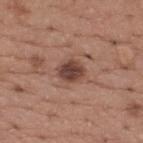The lesion was tiled from a total-body skin photograph and was not biopsied. Located on the upper back. A close-up tile cropped from a whole-body skin photograph, about 15 mm across. A male patient, in their mid-60s.